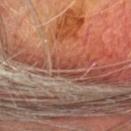Q: Was this lesion biopsied?
A: total-body-photography surveillance lesion; no biopsy
Q: What is the lesion's diameter?
A: ~3.5 mm (longest diameter)
Q: Lesion location?
A: the head or neck
Q: What did automated image analysis measure?
A: an area of roughly 3.5 mm² and a symmetry-axis asymmetry near 0.35; a lesion color around L≈40 a*≈25 b*≈27 in CIELAB, roughly 7 lightness units darker than nearby skin, and a normalized lesion–skin contrast near 6.5
Q: What is the imaging modality?
A: total-body-photography crop, ~15 mm field of view
Q: Who is the patient?
A: male, roughly 65 years of age